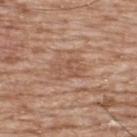follow-up — imaged on a skin check; not biopsied
location — the back
patient — male, approximately 50 years of age
image — total-body-photography crop, ~15 mm field of view
tile lighting — white-light illumination
size — about 4 mm